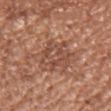Case summary:
* follow-up — imaged on a skin check; not biopsied
* imaging modality — ~15 mm crop, total-body skin-cancer survey
* location — the left upper arm
* patient — female, aged 38 to 42